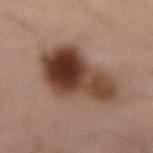Notes:
- site: the lower back
- subject: male, roughly 50 years of age
- acquisition: 15 mm crop, total-body photography
- lesion size: ≈8 mm